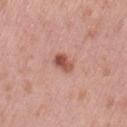Captured during whole-body skin photography for melanoma surveillance; the lesion was not biopsied. On the left thigh. A roughly 15 mm field-of-view crop from a total-body skin photograph. A female subject, aged approximately 55.